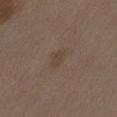- workup — catalogued during a skin exam; not biopsied
- body site — the abdomen
- image source — ~15 mm tile from a whole-body skin photo
- patient — female, aged 33 to 37